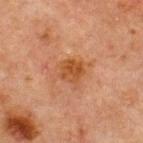follow-up: imaged on a skin check; not biopsied | image: ~15 mm crop, total-body skin-cancer survey | lesion diameter: ≈3 mm | site: the chest | tile lighting: cross-polarized illumination | subject: male, in their mid- to late 60s.